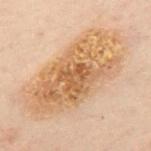This lesion was catalogued during total-body skin photography and was not selected for biopsy.